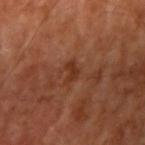Notes:
– workup · no biopsy performed (imaged during a skin exam)
– imaging modality · ~15 mm tile from a whole-body skin photo
– subject · aged 63–67
– location · the right upper arm
– tile lighting · cross-polarized illumination
– lesion diameter · ~2.5 mm (longest diameter)